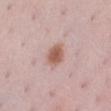This lesion was catalogued during total-body skin photography and was not selected for biopsy. This is a white-light tile. Approximately 3 mm at its widest. A female patient approximately 35 years of age. From the left lower leg. A lesion tile, about 15 mm wide, cut from a 3D total-body photograph.